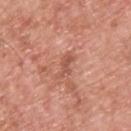Image and clinical context:
A lesion tile, about 15 mm wide, cut from a 3D total-body photograph. Measured at roughly 3 mm in maximum diameter. A male subject in their mid- to late 50s. The tile uses white-light illumination. Located on the upper back.